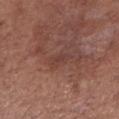The lesion was tiled from a total-body skin photograph and was not biopsied.
Imaged with white-light lighting.
A female subject aged 53–57.
A region of skin cropped from a whole-body photographic capture, roughly 15 mm wide.
On the leg.